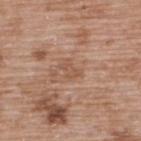  biopsy_status: not biopsied; imaged during a skin examination